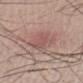{"biopsy_status": "not biopsied; imaged during a skin examination", "lesion_size": {"long_diameter_mm_approx": 6.0}, "patient": {"sex": "male", "age_approx": 50}, "image": {"source": "total-body photography crop", "field_of_view_mm": 15}, "site": "leg"}